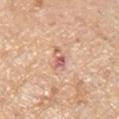Case summary:
– notes · total-body-photography surveillance lesion; no biopsy
– image source · total-body-photography crop, ~15 mm field of view
– patient · male, aged approximately 60
– size · ~3 mm (longest diameter)
– lighting · white-light
– anatomic site · the arm